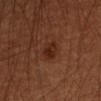Q: What is the imaging modality?
A: total-body-photography crop, ~15 mm field of view
Q: What are the patient's age and sex?
A: male, in their mid-60s
Q: Automated lesion metrics?
A: an average lesion color of about L≈26 a*≈23 b*≈28 (CIELAB) and roughly 7 lightness units darker than nearby skin
Q: What is the anatomic site?
A: the left forearm